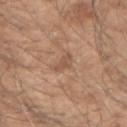workup=catalogued during a skin exam; not biopsied | illumination=white-light illumination | subject=male, in their 50s | image source=~15 mm tile from a whole-body skin photo | location=the right forearm | lesion size=about 3 mm.